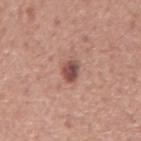{
  "biopsy_status": "not biopsied; imaged during a skin examination",
  "patient": {
    "sex": "male",
    "age_approx": 75
  },
  "lesion_size": {
    "long_diameter_mm_approx": 3.0
  },
  "site": "mid back",
  "lighting": "white-light",
  "image": {
    "source": "total-body photography crop",
    "field_of_view_mm": 15
  }
}